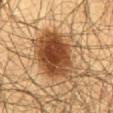  biopsy_status: not biopsied; imaged during a skin examination
  image:
    source: total-body photography crop
    field_of_view_mm: 15
  lesion_size:
    long_diameter_mm_approx: 10.0
  lighting: cross-polarized
  site: abdomen
  patient:
    sex: male
    age_approx: 60